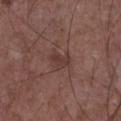{
  "biopsy_status": "not biopsied; imaged during a skin examination",
  "patient": {
    "sex": "male",
    "age_approx": 60
  },
  "image": {
    "source": "total-body photography crop",
    "field_of_view_mm": 15
  },
  "site": "chest",
  "automated_metrics": {
    "eccentricity": 0.75,
    "shape_asymmetry": 0.4,
    "nevus_likeness_0_100": 0,
    "lesion_detection_confidence_0_100": 100
  }
}